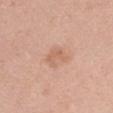Q: Was a biopsy performed?
A: imaged on a skin check; not biopsied
Q: What lighting was used for the tile?
A: white-light
Q: Where on the body is the lesion?
A: the left upper arm
Q: What are the patient's age and sex?
A: male, in their 40s
Q: Automated lesion metrics?
A: a nevus-likeness score of about 0/100 and a detector confidence of about 100 out of 100 that the crop contains a lesion
Q: Lesion size?
A: about 3 mm
Q: What is the imaging modality?
A: total-body-photography crop, ~15 mm field of view